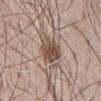Clinical impression: Imaged during a routine full-body skin examination; the lesion was not biopsied and no histopathology is available. Image and clinical context: The lesion is located on the front of the torso. The patient is a male aged approximately 70. A 15 mm close-up extracted from a 3D total-body photography capture. This is a white-light tile.